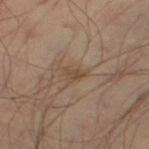Clinical summary:
A male patient, approximately 50 years of age. An algorithmic analysis of the crop reported a nevus-likeness score of about 0/100 and lesion-presence confidence of about 100/100. The lesion is on the leg. A close-up tile cropped from a whole-body skin photograph, about 15 mm across.